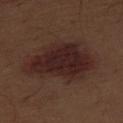No biopsy was performed on this lesion — it was imaged during a full skin examination and was not determined to be concerning. Located on the front of the torso. A male patient roughly 70 years of age. A roughly 15 mm field-of-view crop from a total-body skin photograph.